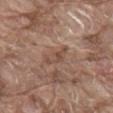Q: Was a biopsy performed?
A: no biopsy performed (imaged during a skin exam)
Q: Where on the body is the lesion?
A: the back
Q: How was the tile lit?
A: white-light
Q: What kind of image is this?
A: ~15 mm tile from a whole-body skin photo
Q: What are the patient's age and sex?
A: male, approximately 80 years of age
Q: What did automated image analysis measure?
A: an average lesion color of about L≈48 a*≈18 b*≈26 (CIELAB) and a normalized border contrast of about 5.5; a border-irregularity rating of about 4.5/10 and radial color variation of about 1.5; a nevus-likeness score of about 0/100 and a lesion-detection confidence of about 75/100
Q: How large is the lesion?
A: about 3.5 mm